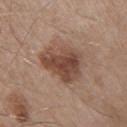Q: Was a biopsy performed?
A: catalogued during a skin exam; not biopsied
Q: Illumination type?
A: white-light
Q: Automated lesion metrics?
A: a footprint of about 17 mm², an outline eccentricity of about 0.75 (0 = round, 1 = elongated), and two-axis asymmetry of about 0.3; a mean CIELAB color near L≈46 a*≈19 b*≈26 and roughly 12 lightness units darker than nearby skin; a border-irregularity rating of about 3/10 and a color-variation rating of about 5/10; a nevus-likeness score of about 70/100
Q: What are the patient's age and sex?
A: male, about 55 years old
Q: Lesion size?
A: ≈6 mm
Q: Lesion location?
A: the right upper arm
Q: What kind of image is this?
A: ~15 mm tile from a whole-body skin photo